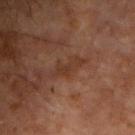Q: Was a biopsy performed?
A: imaged on a skin check; not biopsied
Q: Lesion size?
A: ≈3.5 mm
Q: Patient demographics?
A: male, roughly 70 years of age
Q: Lesion location?
A: the chest
Q: What is the imaging modality?
A: total-body-photography crop, ~15 mm field of view
Q: Illumination type?
A: cross-polarized illumination